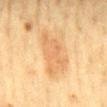Findings:
• follow-up — catalogued during a skin exam; not biopsied
• subject — female, approximately 55 years of age
• acquisition — ~15 mm tile from a whole-body skin photo
• location — the mid back